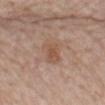No biopsy was performed on this lesion — it was imaged during a full skin examination and was not determined to be concerning. A female subject aged 63–67. The lesion-visualizer software estimated an eccentricity of roughly 0.8 and a shape-asymmetry score of about 0.4 (0 = symmetric). It also reported a lesion color around L≈53 a*≈19 b*≈29 in CIELAB, roughly 7 lightness units darker than nearby skin, and a normalized lesion–skin contrast near 6. The analysis additionally found border irregularity of about 4 on a 0–10 scale, a within-lesion color-variation index near 2.5/10, and a peripheral color-asymmetry measure near 1. The analysis additionally found an automated nevus-likeness rating near 10 out of 100. On the mid back. Longest diameter approximately 3.5 mm. This is a white-light tile. A roughly 15 mm field-of-view crop from a total-body skin photograph.